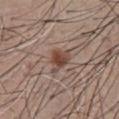<tbp_lesion>
  <biopsy_status>not biopsied; imaged during a skin examination</biopsy_status>
  <site>abdomen</site>
  <image>
    <source>total-body photography crop</source>
    <field_of_view_mm>15</field_of_view_mm>
  </image>
  <lesion_size>
    <long_diameter_mm_approx>3.0</long_diameter_mm_approx>
  </lesion_size>
  <automated_metrics>
    <area_mm2_approx>5.0</area_mm2_approx>
    <eccentricity>0.65</eccentricity>
    <border_irregularity_0_10>4.0</border_irregularity_0_10>
    <color_variation_0_10>3.0</color_variation_0_10>
  </automated_metrics>
  <patient>
    <sex>male</sex>
    <age_approx>55</age_approx>
  </patient>
</tbp_lesion>